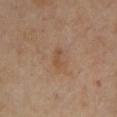Part of a total-body skin-imaging series; this lesion was reviewed on a skin check and was not flagged for biopsy. The total-body-photography lesion software estimated an eccentricity of roughly 0.85 and a symmetry-axis asymmetry near 0.35. The tile uses cross-polarized illumination. A close-up tile cropped from a whole-body skin photograph, about 15 mm across. Approximately 2.5 mm at its widest. A male patient, aged 68–72. On the left upper arm.